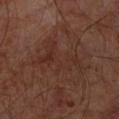follow-up: catalogued during a skin exam; not biopsied | image source: 15 mm crop, total-body photography | patient: male, about 65 years old | location: the left forearm.